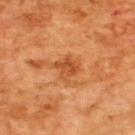Clinical impression: Recorded during total-body skin imaging; not selected for excision or biopsy. Context: Cropped from a total-body skin-imaging series; the visible field is about 15 mm. A male subject in their mid- to late 60s. The lesion is located on the upper back.